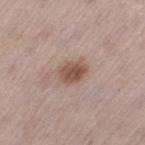Q: Is there a histopathology result?
A: no biopsy performed (imaged during a skin exam)
Q: How was the tile lit?
A: white-light
Q: What is the anatomic site?
A: the left thigh
Q: Who is the patient?
A: male, in their mid-50s
Q: What is the lesion's diameter?
A: ≈3 mm
Q: What kind of image is this?
A: ~15 mm crop, total-body skin-cancer survey
Q: What did automated image analysis measure?
A: an area of roughly 6.5 mm², an outline eccentricity of about 0.65 (0 = round, 1 = elongated), and two-axis asymmetry of about 0.15; a lesion color around L≈52 a*≈18 b*≈26 in CIELAB and roughly 12 lightness units darker than nearby skin; a nevus-likeness score of about 95/100 and a lesion-detection confidence of about 100/100